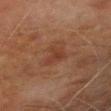{
  "biopsy_status": "not biopsied; imaged during a skin examination",
  "image": {
    "source": "total-body photography crop",
    "field_of_view_mm": 15
  },
  "automated_metrics": {
    "area_mm2_approx": 5.5,
    "eccentricity": 0.75,
    "shape_asymmetry": 0.55,
    "border_irregularity_0_10": 5.5,
    "color_variation_0_10": 2.5,
    "peripheral_color_asymmetry": 0.5,
    "lesion_detection_confidence_0_100": 100
  },
  "patient": {
    "sex": "male",
    "age_approx": 70
  },
  "lighting": "cross-polarized",
  "site": "right forearm"
}